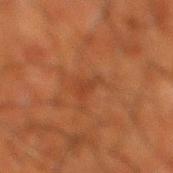Assessment:
The lesion was tiled from a total-body skin photograph and was not biopsied.
Image and clinical context:
Cropped from a whole-body photographic skin survey; the tile spans about 15 mm. On the right lower leg. A male patient in their mid- to late 60s.